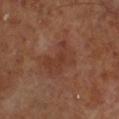workup: no biopsy performed (imaged during a skin exam) | subject: male, aged around 70 | lesion size: ~4.5 mm (longest diameter) | acquisition: 15 mm crop, total-body photography | automated lesion analysis: roughly 6 lightness units darker than nearby skin and a normalized border contrast of about 5.5; internal color variation of about 2 on a 0–10 scale | body site: the right lower leg | illumination: cross-polarized.